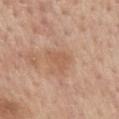The lesion was photographed on a routine skin check and not biopsied; there is no pathology result. A 15 mm close-up extracted from a 3D total-body photography capture. The lesion's longest dimension is about 3 mm. A female subject roughly 65 years of age. The lesion is on the mid back.